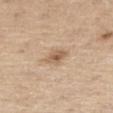workup: catalogued during a skin exam; not biopsied | imaging modality: total-body-photography crop, ~15 mm field of view | patient: male, aged around 70 | TBP lesion metrics: a lesion area of about 5 mm² and an outline eccentricity of about 0.5 (0 = round, 1 = elongated); a lesion color around L≈60 a*≈16 b*≈32 in CIELAB, a lesion–skin lightness drop of about 10, and a normalized border contrast of about 7; an automated nevus-likeness rating near 45 out of 100 and a lesion-detection confidence of about 100/100 | illumination: white-light | anatomic site: the right thigh.